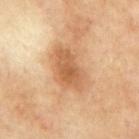follow-up: total-body-photography surveillance lesion; no biopsy | diameter: ~6 mm (longest diameter) | imaging modality: 15 mm crop, total-body photography | tile lighting: cross-polarized illumination | body site: the mid back | patient: male, aged around 70 | automated metrics: a lesion area of about 15 mm², a shape eccentricity near 0.8, and two-axis asymmetry of about 0.25; about 11 CIELAB-L* units darker than the surrounding skin and a lesion-to-skin contrast of about 7.5 (normalized; higher = more distinct); border irregularity of about 3 on a 0–10 scale and a within-lesion color-variation index near 3.5/10; a classifier nevus-likeness of about 50/100.